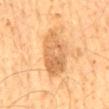Clinical impression:
Recorded during total-body skin imaging; not selected for excision or biopsy.